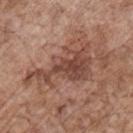The lesion was photographed on a routine skin check and not biopsied; there is no pathology result. The patient is a male in their 70s. The lesion is located on the chest. Cropped from a total-body skin-imaging series; the visible field is about 15 mm.